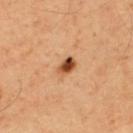<record>
  <biopsy_status>not biopsied; imaged during a skin examination</biopsy_status>
  <site>upper back</site>
  <patient>
    <sex>male</sex>
    <age_approx>50</age_approx>
  </patient>
  <image>
    <source>total-body photography crop</source>
    <field_of_view_mm>15</field_of_view_mm>
  </image>
</record>